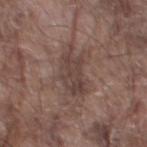notes = catalogued during a skin exam; not biopsied
location = the left thigh
patient = male, about 80 years old
image = total-body-photography crop, ~15 mm field of view
image-analysis metrics = a lesion area of about 10 mm², a shape eccentricity near 0.85, and a symmetry-axis asymmetry near 0.25
lesion diameter = ≈5 mm